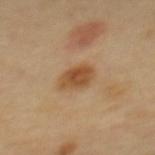Context:
A region of skin cropped from a whole-body photographic capture, roughly 15 mm wide. This is a cross-polarized tile. A female subject about 60 years old. The lesion is located on the upper back.